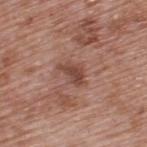Findings:
* workup — total-body-photography surveillance lesion; no biopsy
* tile lighting — white-light
* image source — 15 mm crop, total-body photography
* subject — male, in their 70s
* site — the back